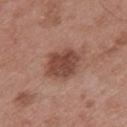Notes:
• notes — imaged on a skin check; not biopsied
• illumination — white-light
• TBP lesion metrics — about 12 CIELAB-L* units darker than the surrounding skin; border irregularity of about 2 on a 0–10 scale, a within-lesion color-variation index near 3/10, and a peripheral color-asymmetry measure near 1; a classifier nevus-likeness of about 45/100 and lesion-presence confidence of about 100/100
• diameter — ≈4 mm
• acquisition — ~15 mm crop, total-body skin-cancer survey
• site — the mid back
• subject — male, aged around 55